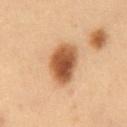No biopsy was performed on this lesion — it was imaged during a full skin examination and was not determined to be concerning. Captured under cross-polarized illumination. Measured at roughly 5 mm in maximum diameter. A male subject, about 55 years old. Located on the abdomen. A roughly 15 mm field-of-view crop from a total-body skin photograph. The lesion-visualizer software estimated an automated nevus-likeness rating near 100 out of 100.